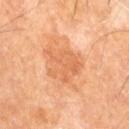Recorded during total-body skin imaging; not selected for excision or biopsy. A 15 mm close-up tile from a total-body photography series done for melanoma screening. From the right leg. A male patient aged 58–62.